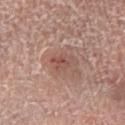Q: Who is the patient?
A: female, aged around 70
Q: How was this image acquired?
A: total-body-photography crop, ~15 mm field of view
Q: What is the anatomic site?
A: the right lower leg